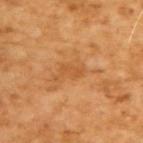The lesion was photographed on a routine skin check and not biopsied; there is no pathology result. Cropped from a total-body skin-imaging series; the visible field is about 15 mm. Automated tile analysis of the lesion measured a lesion color around L≈53 a*≈25 b*≈43 in CIELAB and a normalized border contrast of about 5. A male patient approximately 65 years of age. The tile uses cross-polarized illumination.